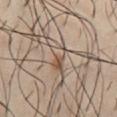Impression:
Captured during whole-body skin photography for melanoma surveillance; the lesion was not biopsied.
Clinical summary:
This is a cross-polarized tile. A male patient about 40 years old. A region of skin cropped from a whole-body photographic capture, roughly 15 mm wide. Measured at roughly 3.5 mm in maximum diameter. Automated image analysis of the tile measured a classifier nevus-likeness of about 5/100 and a detector confidence of about 90 out of 100 that the crop contains a lesion. Located on the abdomen.